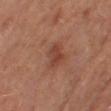Q: Is there a histopathology result?
A: imaged on a skin check; not biopsied
Q: What is the lesion's diameter?
A: ~2.5 mm (longest diameter)
Q: What is the imaging modality?
A: total-body-photography crop, ~15 mm field of view
Q: Patient demographics?
A: female, about 50 years old
Q: Illumination type?
A: cross-polarized
Q: Lesion location?
A: the left thigh